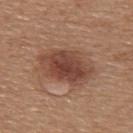The lesion was photographed on a routine skin check and not biopsied; there is no pathology result. Cropped from a total-body skin-imaging series; the visible field is about 15 mm. A female subject in their mid-50s. This is a white-light tile. On the back. The lesion's longest dimension is about 6.5 mm.